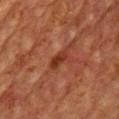Recorded during total-body skin imaging; not selected for excision or biopsy. A male patient aged around 60. The lesion is located on the chest. A 15 mm crop from a total-body photograph taken for skin-cancer surveillance. Captured under cross-polarized illumination.